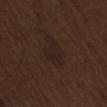notes: catalogued during a skin exam; not biopsied | image source: 15 mm crop, total-body photography | lesion diameter: about 3.5 mm | body site: the lower back | image-analysis metrics: roughly 4 lightness units darker than nearby skin and a lesion-to-skin contrast of about 6 (normalized; higher = more distinct); an automated nevus-likeness rating near 0 out of 100 and lesion-presence confidence of about 100/100 | patient: male, aged around 70.